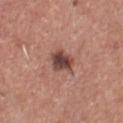| feature | finding |
|---|---|
| diameter | ~3 mm (longest diameter) |
| location | the chest |
| lighting | white-light illumination |
| image source | total-body-photography crop, ~15 mm field of view |
| TBP lesion metrics | a shape eccentricity near 0.5 and a shape-asymmetry score of about 0.3 (0 = symmetric); border irregularity of about 2.5 on a 0–10 scale, a color-variation rating of about 5/10, and peripheral color asymmetry of about 1.5; an automated nevus-likeness rating near 70 out of 100 |
| patient | male, aged 43 to 47 |